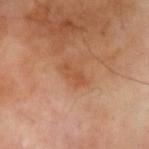Clinical impression:
Part of a total-body skin-imaging series; this lesion was reviewed on a skin check and was not flagged for biopsy.